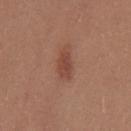workup = imaged on a skin check; not biopsied | body site = the chest | patient = female, aged around 25 | illumination = white-light illumination | image source = ~15 mm crop, total-body skin-cancer survey | lesion diameter = ~3.5 mm (longest diameter).